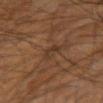Impression: Captured during whole-body skin photography for melanoma surveillance; the lesion was not biopsied. Background: Located on the left forearm. A male patient aged around 60. Cropped from a whole-body photographic skin survey; the tile spans about 15 mm.